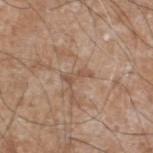– workup: no biopsy performed (imaged during a skin exam)
– body site: the left lower leg
– patient: male, aged approximately 60
– image source: ~15 mm crop, total-body skin-cancer survey
– diameter: ~3 mm (longest diameter)